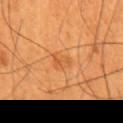The lesion was photographed on a routine skin check and not biopsied; there is no pathology result. A male patient, aged approximately 60. A region of skin cropped from a whole-body photographic capture, roughly 15 mm wide. From the mid back. An algorithmic analysis of the crop reported about 6 CIELAB-L* units darker than the surrounding skin and a lesion-to-skin contrast of about 4.5 (normalized; higher = more distinct).